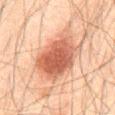The lesion was photographed on a routine skin check and not biopsied; there is no pathology result.
The lesion is located on the abdomen.
The lesion's longest dimension is about 7 mm.
The tile uses cross-polarized illumination.
A close-up tile cropped from a whole-body skin photograph, about 15 mm across.
Automated image analysis of the tile measured a shape eccentricity near 0.75 and a symmetry-axis asymmetry near 0.25.
A male subject in their 60s.